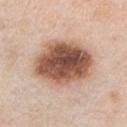The lesion was tiled from a total-body skin photograph and was not biopsied.
The recorded lesion diameter is about 7 mm.
Cropped from a whole-body photographic skin survey; the tile spans about 15 mm.
The tile uses white-light illumination.
On the chest.
A male subject aged approximately 55.